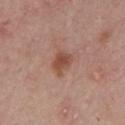follow-up: total-body-photography surveillance lesion; no biopsy | subject: male, approximately 60 years of age | automated metrics: a lesion area of about 5 mm²; an average lesion color of about L≈49 a*≈23 b*≈29 (CIELAB), a lesion–skin lightness drop of about 10, and a lesion-to-skin contrast of about 8 (normalized; higher = more distinct) | diameter: about 3 mm | anatomic site: the chest | image source: total-body-photography crop, ~15 mm field of view | illumination: white-light illumination.